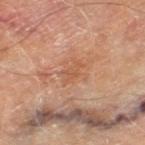Case summary:
– follow-up · catalogued during a skin exam; not biopsied
– image source · ~15 mm crop, total-body skin-cancer survey
– body site · the left thigh
– image-analysis metrics · a footprint of about 3.5 mm², an outline eccentricity of about 0.85 (0 = round, 1 = elongated), and a symmetry-axis asymmetry near 0.4; a nevus-likeness score of about 0/100 and a lesion-detection confidence of about 100/100
– lesion diameter · ≈3 mm
– lighting · cross-polarized illumination
– patient · male, aged approximately 70